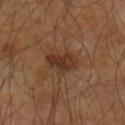Clinical impression: Part of a total-body skin-imaging series; this lesion was reviewed on a skin check and was not flagged for biopsy. Clinical summary: A 15 mm crop from a total-body photograph taken for skin-cancer surveillance. Measured at roughly 3.5 mm in maximum diameter. Located on the arm. A male patient, in their 60s. Imaged with cross-polarized lighting.